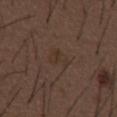Clinical impression: Captured during whole-body skin photography for melanoma surveillance; the lesion was not biopsied. Clinical summary: A region of skin cropped from a whole-body photographic capture, roughly 15 mm wide. A male subject, approximately 50 years of age. The lesion is located on the abdomen.